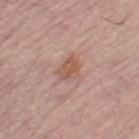body site — the leg | TBP lesion metrics — an average lesion color of about L≈55 a*≈21 b*≈28 (CIELAB), a lesion–skin lightness drop of about 9, and a lesion-to-skin contrast of about 7 (normalized; higher = more distinct); border irregularity of about 2.5 on a 0–10 scale, internal color variation of about 2 on a 0–10 scale, and a peripheral color-asymmetry measure near 1 | subject — female, in their mid-60s | image source — total-body-photography crop, ~15 mm field of view.